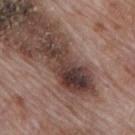biopsy status: imaged on a skin check; not biopsied | imaging modality: 15 mm crop, total-body photography | subject: male, roughly 70 years of age | tile lighting: white-light illumination | diameter: ≈8.5 mm | body site: the mid back | automated lesion analysis: a lesion area of about 19 mm², an outline eccentricity of about 0.95 (0 = round, 1 = elongated), and a symmetry-axis asymmetry near 0.35; a border-irregularity index near 6.5/10, internal color variation of about 8 on a 0–10 scale, and a peripheral color-asymmetry measure near 2.5; an automated nevus-likeness rating near 0 out of 100 and a detector confidence of about 50 out of 100 that the crop contains a lesion.